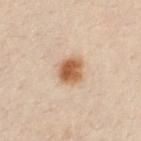Measured at roughly 3 mm in maximum diameter.
From the right upper arm.
This image is a 15 mm lesion crop taken from a total-body photograph.
Captured under cross-polarized illumination.
A male patient, aged 28 to 32.